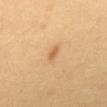The patient is a female aged approximately 55.
Cropped from a total-body skin-imaging series; the visible field is about 15 mm.
The lesion is on the back.